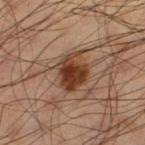This lesion was catalogued during total-body skin photography and was not selected for biopsy. A close-up tile cropped from a whole-body skin photograph, about 15 mm across. The lesion is on the left thigh. A male patient, about 60 years old.